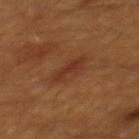Part of a total-body skin-imaging series; this lesion was reviewed on a skin check and was not flagged for biopsy. The patient is a male aged 58–62. A lesion tile, about 15 mm wide, cut from a 3D total-body photograph. Located on the mid back. This is a cross-polarized tile.